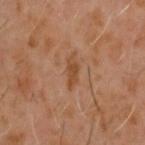Assessment: Captured during whole-body skin photography for melanoma surveillance; the lesion was not biopsied. Clinical summary: A 15 mm crop from a total-body photograph taken for skin-cancer surveillance. From the upper back. A male patient aged approximately 60.